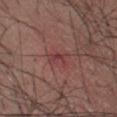Captured during whole-body skin photography for melanoma surveillance; the lesion was not biopsied.
From the left thigh.
Imaged with white-light lighting.
The subject is a male roughly 65 years of age.
A roughly 15 mm field-of-view crop from a total-body skin photograph.